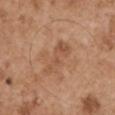{"biopsy_status": "not biopsied; imaged during a skin examination", "patient": {"sex": "male", "age_approx": 55}, "image": {"source": "total-body photography crop", "field_of_view_mm": 15}, "lighting": "white-light", "site": "left upper arm"}